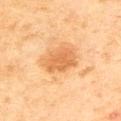Assessment:
Captured during whole-body skin photography for melanoma surveillance; the lesion was not biopsied.
Clinical summary:
This is a cross-polarized tile. The recorded lesion diameter is about 4 mm. Located on the upper back. A 15 mm crop from a total-body photograph taken for skin-cancer surveillance. A male subject aged 43–47.